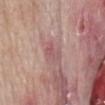Q: Was a biopsy performed?
A: catalogued during a skin exam; not biopsied
Q: Patient demographics?
A: male, roughly 85 years of age or older
Q: Lesion size?
A: about 2.5 mm
Q: Where on the body is the lesion?
A: the mid back
Q: What kind of image is this?
A: ~15 mm tile from a whole-body skin photo
Q: What lighting was used for the tile?
A: white-light illumination